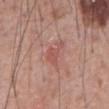<tbp_lesion>
  <biopsy_status>not biopsied; imaged during a skin examination</biopsy_status>
  <site>front of the torso</site>
  <patient>
    <sex>male</sex>
    <age_approx>60</age_approx>
  </patient>
  <image>
    <source>total-body photography crop</source>
    <field_of_view_mm>15</field_of_view_mm>
  </image>
  <lesion_size>
    <long_diameter_mm_approx>3.5</long_diameter_mm_approx>
  </lesion_size>
  <automated_metrics>
    <area_mm2_approx>4.5</area_mm2_approx>
    <eccentricity>0.8</eccentricity>
    <shape_asymmetry>0.6</shape_asymmetry>
    <cielab_L>53</cielab_L>
    <cielab_a>26</cielab_a>
    <cielab_b>25</cielab_b>
    <vs_skin_darker_L>7.0</vs_skin_darker_L>
    <vs_skin_contrast_norm>5.0</vs_skin_contrast_norm>
  </automated_metrics>
</tbp_lesion>